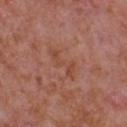Impression:
Captured during whole-body skin photography for melanoma surveillance; the lesion was not biopsied.
Acquisition and patient details:
A close-up tile cropped from a whole-body skin photograph, about 15 mm across. The recorded lesion diameter is about 4.5 mm. The tile uses white-light illumination. The total-body-photography lesion software estimated an area of roughly 5.5 mm² and an outline eccentricity of about 0.95 (0 = round, 1 = elongated). It also reported an automated nevus-likeness rating near 0 out of 100. A male patient aged around 65. Located on the chest.